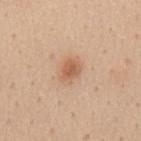{"biopsy_status": "not biopsied; imaged during a skin examination", "patient": {"sex": "female", "age_approx": 45}, "site": "mid back", "lesion_size": {"long_diameter_mm_approx": 3.0}, "image": {"source": "total-body photography crop", "field_of_view_mm": 15}}